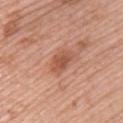Impression:
The lesion was tiled from a total-body skin photograph and was not biopsied.
Background:
A female subject, aged approximately 60. The tile uses white-light illumination. The lesion is located on the upper back. Cropped from a total-body skin-imaging series; the visible field is about 15 mm. Measured at roughly 3.5 mm in maximum diameter.